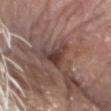  biopsy_status: not biopsied; imaged during a skin examination